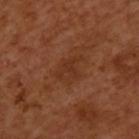About 4 mm across. This is a cross-polarized tile. The patient is a male about 50 years old. From the upper back. Automated image analysis of the tile measured a border-irregularity index near 3.5/10, a color-variation rating of about 2/10, and a peripheral color-asymmetry measure near 0.5. A 15 mm close-up tile from a total-body photography series done for melanoma screening.